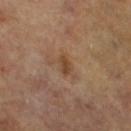follow-up: total-body-photography surveillance lesion; no biopsy
size: about 2.5 mm
anatomic site: the leg
subject: female, roughly 65 years of age
illumination: cross-polarized illumination
acquisition: 15 mm crop, total-body photography
image-analysis metrics: an area of roughly 2.5 mm², an outline eccentricity of about 0.9 (0 = round, 1 = elongated), and two-axis asymmetry of about 0.35; a border-irregularity index near 3/10, internal color variation of about 0.5 on a 0–10 scale, and radial color variation of about 0; an automated nevus-likeness rating near 5 out of 100 and lesion-presence confidence of about 100/100